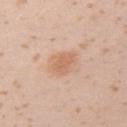<lesion>
<biopsy_status>not biopsied; imaged during a skin examination</biopsy_status>
<image>
  <source>total-body photography crop</source>
  <field_of_view_mm>15</field_of_view_mm>
</image>
<patient>
  <sex>female</sex>
  <age_approx>20</age_approx>
</patient>
<lighting>white-light</lighting>
<site>left upper arm</site>
<lesion_size>
  <long_diameter_mm_approx>3.5</long_diameter_mm_approx>
</lesion_size>
</lesion>